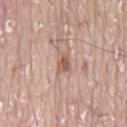No biopsy was performed on this lesion — it was imaged during a full skin examination and was not determined to be concerning. A 15 mm crop from a total-body photograph taken for skin-cancer surveillance. A male patient aged 73–77. From the back. Captured under white-light illumination. Approximately 2.5 mm at its widest. An algorithmic analysis of the crop reported an eccentricity of roughly 0.75 and a shape-asymmetry score of about 0.35 (0 = symmetric). The software also gave a lesion-to-skin contrast of about 7.5 (normalized; higher = more distinct). The analysis additionally found a nevus-likeness score of about 0/100.